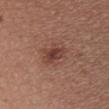The lesion was tiled from a total-body skin photograph and was not biopsied. The tile uses white-light illumination. The lesion's longest dimension is about 4 mm. A 15 mm close-up tile from a total-body photography series done for melanoma screening. The patient is a male approximately 40 years of age. From the chest.